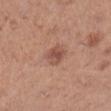Recorded during total-body skin imaging; not selected for excision or biopsy. The lesion is on the right lower leg. A region of skin cropped from a whole-body photographic capture, roughly 15 mm wide. A female subject, aged 28–32. The lesion's longest dimension is about 2.5 mm.